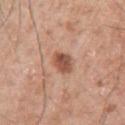The lesion-visualizer software estimated an area of roughly 5 mm², an eccentricity of roughly 0.65, and a symmetry-axis asymmetry near 0.25. The analysis additionally found a lesion color around L≈52 a*≈23 b*≈30 in CIELAB, a lesion–skin lightness drop of about 13, and a lesion-to-skin contrast of about 9 (normalized; higher = more distinct). And it measured a border-irregularity index near 2/10, a within-lesion color-variation index near 4/10, and a peripheral color-asymmetry measure near 1. The software also gave an automated nevus-likeness rating near 90 out of 100 and a lesion-detection confidence of about 100/100. A close-up tile cropped from a whole-body skin photograph, about 15 mm across. The lesion is on the left arm. Captured under white-light illumination. The patient is a male aged approximately 70. The lesion's longest dimension is about 3 mm.